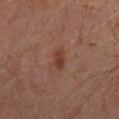Clinical impression: The lesion was tiled from a total-body skin photograph and was not biopsied. Acquisition and patient details: Cropped from a total-body skin-imaging series; the visible field is about 15 mm. Measured at roughly 3 mm in maximum diameter. This is a cross-polarized tile. From the mid back. The patient is a male approximately 60 years of age.